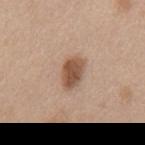Imaged during a routine full-body skin examination; the lesion was not biopsied and no histopathology is available.
A female patient about 40 years old.
Imaged with white-light lighting.
The recorded lesion diameter is about 3.5 mm.
This image is a 15 mm lesion crop taken from a total-body photograph.
On the mid back.